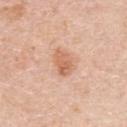Image and clinical context: Captured under white-light illumination. Approximately 3.5 mm at its widest. Located on the upper back. The subject is a female about 50 years old. A region of skin cropped from a whole-body photographic capture, roughly 15 mm wide.